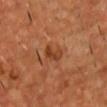follow-up — no biopsy performed (imaged during a skin exam); image-analysis metrics — an average lesion color of about L≈37 a*≈24 b*≈34 (CIELAB) and about 8 CIELAB-L* units darker than the surrounding skin; acquisition — 15 mm crop, total-body photography; anatomic site — the chest; tile lighting — cross-polarized; diameter — ≈3 mm; patient — male, in their mid-50s.